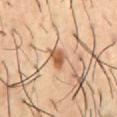follow-up: no biopsy performed (imaged during a skin exam) | subject: male, approximately 55 years of age | image source: 15 mm crop, total-body photography | automated lesion analysis: an area of roughly 4 mm² and a shape-asymmetry score of about 0.3 (0 = symmetric); a lesion-to-skin contrast of about 9.5 (normalized; higher = more distinct) | location: the abdomen | tile lighting: cross-polarized | lesion diameter: ≈2.5 mm.